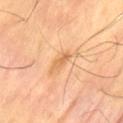Findings:
- follow-up — total-body-photography surveillance lesion; no biopsy
- acquisition — ~15 mm tile from a whole-body skin photo
- TBP lesion metrics — a lesion area of about 3 mm², an outline eccentricity of about 0.85 (0 = round, 1 = elongated), and two-axis asymmetry of about 0.3
- patient — male, approximately 60 years of age
- lighting — cross-polarized illumination
- anatomic site — the lower back
- lesion diameter — about 3 mm